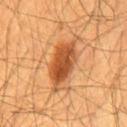<tbp_lesion>
  <biopsy_status>not biopsied; imaged during a skin examination</biopsy_status>
  <image>
    <source>total-body photography crop</source>
    <field_of_view_mm>15</field_of_view_mm>
  </image>
  <lesion_size>
    <long_diameter_mm_approx>7.0</long_diameter_mm_approx>
  </lesion_size>
  <site>chest</site>
  <patient>
    <sex>male</sex>
    <age_approx>60</age_approx>
  </patient>
</tbp_lesion>